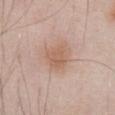Q: How was this image acquired?
A: ~15 mm tile from a whole-body skin photo
Q: Lesion location?
A: the abdomen
Q: What are the patient's age and sex?
A: male, roughly 55 years of age
Q: What is the lesion's diameter?
A: about 3 mm
Q: What lighting was used for the tile?
A: white-light illumination